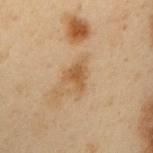workup: total-body-photography surveillance lesion; no biopsy | subject: male, roughly 55 years of age | acquisition: ~15 mm tile from a whole-body skin photo | body site: the left upper arm | tile lighting: cross-polarized | lesion size: about 3 mm | image-analysis metrics: a footprint of about 4.5 mm², an eccentricity of roughly 0.55, and a shape-asymmetry score of about 0.5 (0 = symmetric); a mean CIELAB color near L≈44 a*≈15 b*≈32, a lesion–skin lightness drop of about 8, and a normalized lesion–skin contrast near 7.5; a lesion-detection confidence of about 100/100.